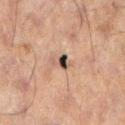Assessment: This lesion was catalogued during total-body skin photography and was not selected for biopsy. Background: A male subject in their 60s. On the left lower leg. A lesion tile, about 15 mm wide, cut from a 3D total-body photograph. Captured under cross-polarized illumination. Automated tile analysis of the lesion measured a lesion area of about 3 mm², an eccentricity of roughly 0.8, and two-axis asymmetry of about 0.3. The software also gave a border-irregularity rating of about 3/10, a within-lesion color-variation index near 6.5/10, and radial color variation of about 2. The analysis additionally found a classifier nevus-likeness of about 0/100 and lesion-presence confidence of about 100/100.